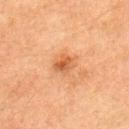The lesion was photographed on a routine skin check and not biopsied; there is no pathology result. A 15 mm close-up extracted from a 3D total-body photography capture. Located on the left upper arm. Longest diameter approximately 2.5 mm. The patient is a male roughly 75 years of age. This is a cross-polarized tile.